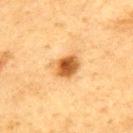Findings:
- biopsy status · no biopsy performed (imaged during a skin exam)
- subject · male, in their mid- to late 70s
- lesion size · ≈3.5 mm
- illumination · cross-polarized illumination
- automated metrics · a lesion area of about 7.5 mm², an eccentricity of roughly 0.6, and a symmetry-axis asymmetry near 0.2; a classifier nevus-likeness of about 100/100 and a lesion-detection confidence of about 100/100
- imaging modality · ~15 mm crop, total-body skin-cancer survey
- body site · the mid back